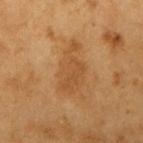Captured during whole-body skin photography for melanoma surveillance; the lesion was not biopsied. Imaged with cross-polarized lighting. From the right upper arm. A 15 mm crop from a total-body photograph taken for skin-cancer surveillance. The subject is a male aged 58–62. Automated image analysis of the tile measured a lesion–skin lightness drop of about 7 and a normalized border contrast of about 5.5. The analysis additionally found a border-irregularity rating of about 4.5/10, a color-variation rating of about 2/10, and radial color variation of about 0.5. It also reported an automated nevus-likeness rating near 0 out of 100 and a lesion-detection confidence of about 100/100.